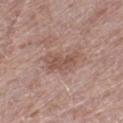Q: Is there a histopathology result?
A: total-body-photography surveillance lesion; no biopsy
Q: Lesion size?
A: ~4 mm (longest diameter)
Q: What are the patient's age and sex?
A: male, roughly 70 years of age
Q: What is the anatomic site?
A: the left thigh
Q: What kind of image is this?
A: 15 mm crop, total-body photography
Q: Illumination type?
A: white-light